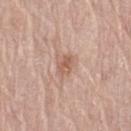This lesion was catalogued during total-body skin photography and was not selected for biopsy.